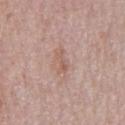Findings:
* follow-up: total-body-photography surveillance lesion; no biopsy
* lesion size: ≈2.5 mm
* automated lesion analysis: internal color variation of about 0 on a 0–10 scale and a peripheral color-asymmetry measure near 0
* patient: male, aged 68–72
* image: 15 mm crop, total-body photography
* site: the abdomen